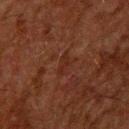| feature | finding |
|---|---|
| biopsy status | total-body-photography surveillance lesion; no biopsy |
| tile lighting | cross-polarized illumination |
| lesion size | ≈3 mm |
| subject | male, in their 60s |
| anatomic site | the upper back |
| imaging modality | total-body-photography crop, ~15 mm field of view |